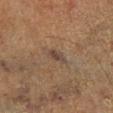Image and clinical context: A region of skin cropped from a whole-body photographic capture, roughly 15 mm wide. The patient is a male aged 68 to 72. The lesion is located on the leg. The lesion's longest dimension is about 2.5 mm. Captured under cross-polarized illumination.